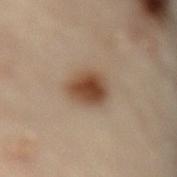Captured during whole-body skin photography for melanoma surveillance; the lesion was not biopsied. A 15 mm crop from a total-body photograph taken for skin-cancer surveillance. About 4 mm across. Located on the back. The total-body-photography lesion software estimated an area of roughly 9.5 mm², a shape eccentricity near 0.6, and two-axis asymmetry of about 0.15. And it measured border irregularity of about 1.5 on a 0–10 scale, internal color variation of about 5 on a 0–10 scale, and peripheral color asymmetry of about 1.5. The analysis additionally found a lesion-detection confidence of about 100/100. This is a cross-polarized tile. A female subject, aged approximately 60.